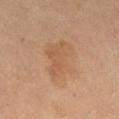A 15 mm close-up tile from a total-body photography series done for melanoma screening. The lesion is located on the right thigh. This is a cross-polarized tile. Measured at roughly 5.5 mm in maximum diameter. A female patient, in their 70s.